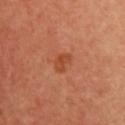biopsy status: no biopsy performed (imaged during a skin exam) | TBP lesion metrics: a lesion area of about 3 mm², an outline eccentricity of about 0.8 (0 = round, 1 = elongated), and a shape-asymmetry score of about 0.35 (0 = symmetric); a border-irregularity index near 3.5/10, a within-lesion color-variation index near 1/10, and a peripheral color-asymmetry measure near 0.5; a nevus-likeness score of about 10/100 | illumination: cross-polarized illumination | size: ≈2.5 mm | imaging modality: 15 mm crop, total-body photography | subject: female, in their 50s.